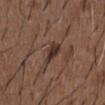{"biopsy_status": "not biopsied; imaged during a skin examination", "image": {"source": "total-body photography crop", "field_of_view_mm": 15}, "patient": {"sex": "male", "age_approx": 50}, "lighting": "white-light", "lesion_size": {"long_diameter_mm_approx": 4.0}, "automated_metrics": {"cielab_L": 33, "cielab_a": 16, "cielab_b": 21, "vs_skin_darker_L": 10.0, "lesion_detection_confidence_0_100": 90}, "site": "chest"}